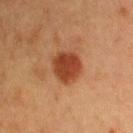A female patient roughly 50 years of age. On the right upper arm. Captured under cross-polarized illumination. Longest diameter approximately 4 mm. A 15 mm close-up extracted from a 3D total-body photography capture.